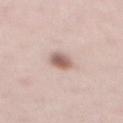Findings:
- body site · the front of the torso
- subject · male, in their 40s
- imaging modality · ~15 mm crop, total-body skin-cancer survey